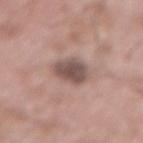notes: no biopsy performed (imaged during a skin exam); imaging modality: total-body-photography crop, ~15 mm field of view; subject: male, approximately 55 years of age; diameter: about 3.5 mm; lighting: white-light; location: the lower back.